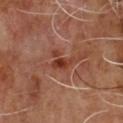Assessment: No biopsy was performed on this lesion — it was imaged during a full skin examination and was not determined to be concerning. Acquisition and patient details: The patient is a male in their mid-60s. A 15 mm close-up tile from a total-body photography series done for melanoma screening. Measured at roughly 3.5 mm in maximum diameter. The lesion is on the chest. Automated image analysis of the tile measured border irregularity of about 6 on a 0–10 scale, a color-variation rating of about 3.5/10, and radial color variation of about 1. It also reported a classifier nevus-likeness of about 0/100.